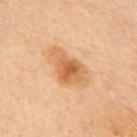Captured during whole-body skin photography for melanoma surveillance; the lesion was not biopsied. A male patient, approximately 70 years of age. The total-body-photography lesion software estimated an average lesion color of about L≈51 a*≈19 b*≈34 (CIELAB), a lesion–skin lightness drop of about 9, and a normalized border contrast of about 7.5. And it measured a nevus-likeness score of about 65/100 and a detector confidence of about 100 out of 100 that the crop contains a lesion. From the chest. This is a cross-polarized tile. A lesion tile, about 15 mm wide, cut from a 3D total-body photograph.